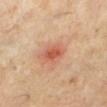Assessment:
Part of a total-body skin-imaging series; this lesion was reviewed on a skin check and was not flagged for biopsy.
Background:
The subject is a female aged 38 to 42. This is a cross-polarized tile. This image is a 15 mm lesion crop taken from a total-body photograph. On the left lower leg. About 3 mm across.